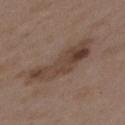The lesion was photographed on a routine skin check and not biopsied; there is no pathology result. A close-up tile cropped from a whole-body skin photograph, about 15 mm across. A female patient aged approximately 35. An algorithmic analysis of the crop reported an average lesion color of about L≈42 a*≈15 b*≈24 (CIELAB), roughly 9 lightness units darker than nearby skin, and a normalized lesion–skin contrast near 7.5. The analysis additionally found a detector confidence of about 100 out of 100 that the crop contains a lesion. Located on the back. Captured under white-light illumination.